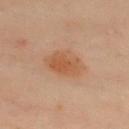| feature | finding |
|---|---|
| patient | female, approximately 60 years of age |
| image | 15 mm crop, total-body photography |
| site | the chest |
| lighting | cross-polarized |
| lesion diameter | ≈5 mm |